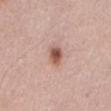The lesion was photographed on a routine skin check and not biopsied; there is no pathology result. Automated tile analysis of the lesion measured a lesion color around L≈55 a*≈22 b*≈27 in CIELAB, roughly 14 lightness units darker than nearby skin, and a lesion-to-skin contrast of about 9.5 (normalized; higher = more distinct). The software also gave a border-irregularity index near 2/10 and internal color variation of about 5.5 on a 0–10 scale. The analysis additionally found a detector confidence of about 100 out of 100 that the crop contains a lesion. Cropped from a total-body skin-imaging series; the visible field is about 15 mm. A male patient approximately 65 years of age. Imaged with white-light lighting. The lesion is on the mid back. Approximately 3 mm at its widest.